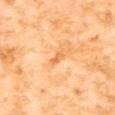The lesion is on the upper back. The subject is a female aged around 55. A roughly 15 mm field-of-view crop from a total-body skin photograph. The total-body-photography lesion software estimated roughly 9 lightness units darker than nearby skin and a normalized lesion–skin contrast near 6. It also reported a within-lesion color-variation index near 0/10 and radial color variation of about 0. Imaged with cross-polarized lighting.